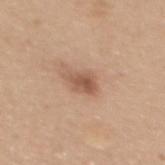Assessment: The lesion was photographed on a routine skin check and not biopsied; there is no pathology result. Image and clinical context: From the mid back. This image is a 15 mm lesion crop taken from a total-body photograph. The subject is a male aged around 40. This is a white-light tile. The lesion's longest dimension is about 2.5 mm. The total-body-photography lesion software estimated a lesion area of about 4.5 mm², an eccentricity of roughly 0.65, and a symmetry-axis asymmetry near 0.3. The software also gave an average lesion color of about L≈55 a*≈20 b*≈31 (CIELAB), roughly 11 lightness units darker than nearby skin, and a normalized lesion–skin contrast near 7.5. And it measured a classifier nevus-likeness of about 65/100 and a detector confidence of about 100 out of 100 that the crop contains a lesion.